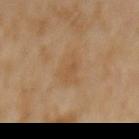notes: no biopsy performed (imaged during a skin exam)
subject: male, aged around 60
lesion diameter: about 4 mm
location: the abdomen
acquisition: 15 mm crop, total-body photography
TBP lesion metrics: an area of roughly 8 mm² and a symmetry-axis asymmetry near 0.25; a mean CIELAB color near L≈50 a*≈16 b*≈34, roughly 5 lightness units darker than nearby skin, and a normalized border contrast of about 4.5
illumination: cross-polarized illumination